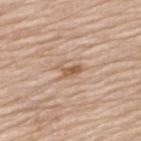Captured during whole-body skin photography for melanoma surveillance; the lesion was not biopsied.
Imaged with white-light lighting.
Automated tile analysis of the lesion measured a footprint of about 3.5 mm², a shape eccentricity near 0.8, and a shape-asymmetry score of about 0.5 (0 = symmetric). The software also gave an average lesion color of about L≈58 a*≈19 b*≈31 (CIELAB), a lesion–skin lightness drop of about 10, and a lesion-to-skin contrast of about 7 (normalized; higher = more distinct). The software also gave a border-irregularity index near 5/10, a within-lesion color-variation index near 2.5/10, and a peripheral color-asymmetry measure near 1. The analysis additionally found a nevus-likeness score of about 0/100 and a detector confidence of about 100 out of 100 that the crop contains a lesion.
The subject is a male in their mid- to late 70s.
A roughly 15 mm field-of-view crop from a total-body skin photograph.
The lesion is on the upper back.